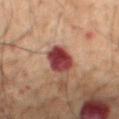Recorded during total-body skin imaging; not selected for excision or biopsy. The total-body-photography lesion software estimated a shape eccentricity near 0.45 and a shape-asymmetry score of about 0.15 (0 = symmetric). It also reported roughly 16 lightness units darker than nearby skin and a normalized lesion–skin contrast near 15. It also reported border irregularity of about 1.5 on a 0–10 scale and a peripheral color-asymmetry measure near 1.5. The analysis additionally found a nevus-likeness score of about 0/100 and lesion-presence confidence of about 100/100. From the mid back. The lesion's longest dimension is about 3.5 mm. Imaged with cross-polarized lighting. Cropped from a whole-body photographic skin survey; the tile spans about 15 mm. A male patient, aged 48 to 52.